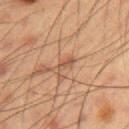{"biopsy_status": "not biopsied; imaged during a skin examination", "lighting": "cross-polarized", "site": "right upper arm", "image": {"source": "total-body photography crop", "field_of_view_mm": 15}, "patient": {"sex": "male", "age_approx": 55}, "lesion_size": {"long_diameter_mm_approx": 3.0}}